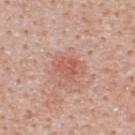Captured during whole-body skin photography for melanoma surveillance; the lesion was not biopsied. About 3 mm across. Captured under white-light illumination. On the upper back. The total-body-photography lesion software estimated a border-irregularity index near 3.5/10, a color-variation rating of about 2.5/10, and a peripheral color-asymmetry measure near 1. A male patient aged approximately 40. A roughly 15 mm field-of-view crop from a total-body skin photograph.